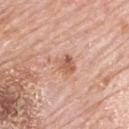No biopsy was performed on this lesion — it was imaged during a full skin examination and was not determined to be concerning. This is a white-light tile. Measured at roughly 3.5 mm in maximum diameter. On the mid back. A male subject approximately 80 years of age. A lesion tile, about 15 mm wide, cut from a 3D total-body photograph.